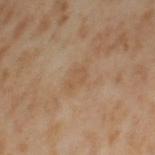Impression: The lesion was tiled from a total-body skin photograph and was not biopsied. Image and clinical context: From the left thigh. A female subject, aged approximately 55. The lesion's longest dimension is about 3 mm. A region of skin cropped from a whole-body photographic capture, roughly 15 mm wide. Imaged with cross-polarized lighting.